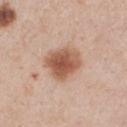No biopsy was performed on this lesion — it was imaged during a full skin examination and was not determined to be concerning. The lesion is on the chest. A close-up tile cropped from a whole-body skin photograph, about 15 mm across. The tile uses white-light illumination. A male patient, aged 58 to 62. Approximately 4.5 mm at its widest.